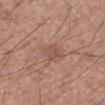Q: Was this lesion biopsied?
A: total-body-photography surveillance lesion; no biopsy